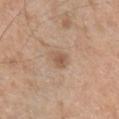lesion size: about 2.5 mm
image source: ~15 mm tile from a whole-body skin photo
patient: male, aged 53–57
tile lighting: white-light
site: the chest